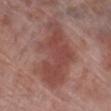The lesion was photographed on a routine skin check and not biopsied; there is no pathology result.
From the leg.
This is a white-light tile.
Longest diameter approximately 9 mm.
A male subject in their 70s.
The total-body-photography lesion software estimated an area of roughly 29 mm², an outline eccentricity of about 0.85 (0 = round, 1 = elongated), and a shape-asymmetry score of about 0.3 (0 = symmetric). It also reported a classifier nevus-likeness of about 50/100 and a lesion-detection confidence of about 100/100.
A 15 mm close-up tile from a total-body photography series done for melanoma screening.